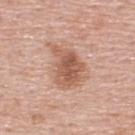Clinical impression:
The lesion was photographed on a routine skin check and not biopsied; there is no pathology result.
Acquisition and patient details:
This is a white-light tile. From the upper back. A 15 mm close-up tile from a total-body photography series done for melanoma screening. The subject is a male aged 53 to 57.